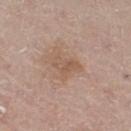This lesion was catalogued during total-body skin photography and was not selected for biopsy. The recorded lesion diameter is about 3.5 mm. A male subject, approximately 70 years of age. The lesion is located on the left leg. Cropped from a total-body skin-imaging series; the visible field is about 15 mm. This is a white-light tile.